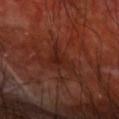workup: total-body-photography surveillance lesion; no biopsy
image: 15 mm crop, total-body photography
TBP lesion metrics: a lesion color around L≈24 a*≈24 b*≈25 in CIELAB, roughly 5 lightness units darker than nearby skin, and a lesion-to-skin contrast of about 6 (normalized; higher = more distinct)
illumination: cross-polarized
site: the right upper arm
lesion diameter: ≈4 mm
patient: male, aged approximately 60